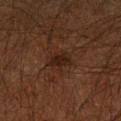| feature | finding |
|---|---|
| biopsy status | no biopsy performed (imaged during a skin exam) |
| patient | male, aged 63 to 67 |
| lighting | cross-polarized illumination |
| size | about 2.5 mm |
| site | the arm |
| image | ~15 mm crop, total-body skin-cancer survey |